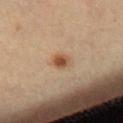Findings:
* biopsy status — total-body-photography surveillance lesion; no biopsy
* illumination — cross-polarized
* lesion size — ≈2.5 mm
* patient — female, aged 43–47
* anatomic site — the chest
* image source — total-body-photography crop, ~15 mm field of view